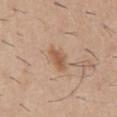Notes:
* follow-up: no biopsy performed (imaged during a skin exam)
* patient: male, approximately 30 years of age
* lesion size: ~3.5 mm (longest diameter)
* site: the abdomen
* imaging modality: ~15 mm crop, total-body skin-cancer survey
* lighting: white-light
* automated metrics: a footprint of about 5.5 mm², an outline eccentricity of about 0.85 (0 = round, 1 = elongated), and a symmetry-axis asymmetry near 0.25; an average lesion color of about L≈57 a*≈20 b*≈33 (CIELAB), about 10 CIELAB-L* units darker than the surrounding skin, and a normalized lesion–skin contrast near 7; a border-irregularity index near 2.5/10, a color-variation rating of about 2/10, and a peripheral color-asymmetry measure near 0.5